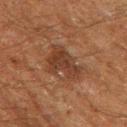Clinical impression: This lesion was catalogued during total-body skin photography and was not selected for biopsy. Background: From the left thigh. The recorded lesion diameter is about 4.5 mm. A roughly 15 mm field-of-view crop from a total-body skin photograph. Captured under cross-polarized illumination. The lesion-visualizer software estimated a within-lesion color-variation index near 2.5/10 and a peripheral color-asymmetry measure near 1. The software also gave a lesion-detection confidence of about 100/100. A male patient, aged around 60.